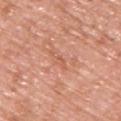biopsy_status: not biopsied; imaged during a skin examination
image:
  source: total-body photography crop
  field_of_view_mm: 15
lighting: white-light
lesion_size:
  long_diameter_mm_approx: 3.0
automated_metrics:
  area_mm2_approx: 2.0
  shape_asymmetry: 0.3
  border_irregularity_0_10: 4.0
  color_variation_0_10: 0.0
  peripheral_color_asymmetry: 0.0
site: upper back
patient:
  sex: male
  age_approx: 60